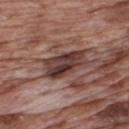lesion diameter — ≈4.5 mm; subject — male, aged 68–72; acquisition — 15 mm crop, total-body photography; lighting — white-light illumination; anatomic site — the upper back.